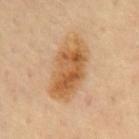follow-up: imaged on a skin check; not biopsied
subject: male, aged approximately 70
acquisition: 15 mm crop, total-body photography
location: the mid back